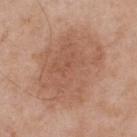This lesion was catalogued during total-body skin photography and was not selected for biopsy.
Captured under white-light illumination.
Automated image analysis of the tile measured a footprint of about 55 mm², a shape eccentricity near 0.65, and a symmetry-axis asymmetry near 0.15.
Cropped from a total-body skin-imaging series; the visible field is about 15 mm.
A male patient, about 55 years old.
Located on the back.
About 10.5 mm across.